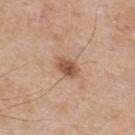The lesion was tiled from a total-body skin photograph and was not biopsied.
A lesion tile, about 15 mm wide, cut from a 3D total-body photograph.
On the back.
A male patient approximately 55 years of age.